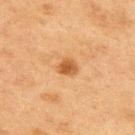Image and clinical context:
Longest diameter approximately 2.5 mm. The tile uses cross-polarized illumination. A 15 mm close-up extracted from a 3D total-body photography capture. On the back. Automated image analysis of the tile measured a mean CIELAB color near L≈46 a*≈21 b*≈37, a lesion–skin lightness drop of about 10, and a normalized border contrast of about 8. And it measured a border-irregularity index near 2/10, a within-lesion color-variation index near 2/10, and radial color variation of about 0.5. The software also gave an automated nevus-likeness rating near 95 out of 100 and a detector confidence of about 100 out of 100 that the crop contains a lesion. The subject is a male in their mid-70s.